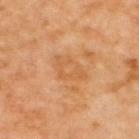{"biopsy_status": "not biopsied; imaged during a skin examination", "image": {"source": "total-body photography crop", "field_of_view_mm": 15}, "patient": {"age_approx": 65}, "lesion_size": {"long_diameter_mm_approx": 4.5}, "automated_metrics": {"eccentricity": 0.8, "shape_asymmetry": 0.35, "border_irregularity_0_10": 3.5, "color_variation_0_10": 3.0, "nevus_likeness_0_100": 0, "lesion_detection_confidence_0_100": 100}, "site": "upper back"}